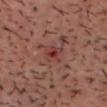Q: Is there a histopathology result?
A: catalogued during a skin exam; not biopsied
Q: What are the patient's age and sex?
A: male, approximately 40 years of age
Q: What lighting was used for the tile?
A: cross-polarized
Q: Lesion size?
A: ~3 mm (longest diameter)
Q: What kind of image is this?
A: ~15 mm tile from a whole-body skin photo
Q: Automated lesion metrics?
A: a footprint of about 5.5 mm², an eccentricity of roughly 0.6, and two-axis asymmetry of about 0.3; a border-irregularity rating of about 3.5/10, internal color variation of about 8.5 on a 0–10 scale, and peripheral color asymmetry of about 2.5; an automated nevus-likeness rating near 0 out of 100 and lesion-presence confidence of about 100/100
Q: Lesion location?
A: the head or neck